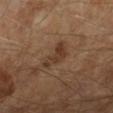{
  "biopsy_status": "not biopsied; imaged during a skin examination",
  "patient": {
    "sex": "male",
    "age_approx": 65
  },
  "image": {
    "source": "total-body photography crop",
    "field_of_view_mm": 15
  },
  "lighting": "cross-polarized"
}